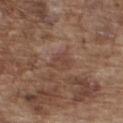Part of a total-body skin-imaging series; this lesion was reviewed on a skin check and was not flagged for biopsy. The subject is a male in their mid- to late 70s. The lesion is on the chest. A close-up tile cropped from a whole-body skin photograph, about 15 mm across.